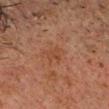Recorded during total-body skin imaging; not selected for excision or biopsy. Cropped from a whole-body photographic skin survey; the tile spans about 15 mm. This is a cross-polarized tile. The lesion is located on the head or neck. A male patient, aged 58–62.